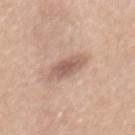tile lighting = white-light | patient = female, aged 28 to 32 | image source = ~15 mm tile from a whole-body skin photo | body site = the mid back.